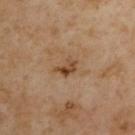Q: Was a biopsy performed?
A: imaged on a skin check; not biopsied
Q: How large is the lesion?
A: ≈3 mm
Q: Patient demographics?
A: male, roughly 55 years of age
Q: What is the imaging modality?
A: total-body-photography crop, ~15 mm field of view
Q: How was the tile lit?
A: cross-polarized illumination
Q: Where on the body is the lesion?
A: the left upper arm
Q: What did automated image analysis measure?
A: a lesion area of about 3.5 mm² and an outline eccentricity of about 0.75 (0 = round, 1 = elongated); a border-irregularity rating of about 4.5/10 and peripheral color asymmetry of about 2.5